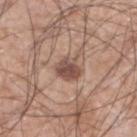location — the left lower leg
image — ~15 mm crop, total-body skin-cancer survey
patient — male, aged around 60